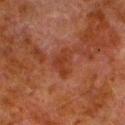{
  "biopsy_status": "not biopsied; imaged during a skin examination",
  "image": {
    "source": "total-body photography crop",
    "field_of_view_mm": 15
  },
  "patient": {
    "sex": "male",
    "age_approx": 80
  },
  "site": "left lower leg",
  "lighting": "cross-polarized",
  "automated_metrics": {
    "area_mm2_approx": 5.5,
    "eccentricity": 0.85,
    "shape_asymmetry": 0.5,
    "border_irregularity_0_10": 5.0,
    "nevus_likeness_0_100": 0,
    "lesion_detection_confidence_0_100": 100
  }
}